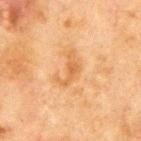The lesion was photographed on a routine skin check and not biopsied; there is no pathology result.
The lesion is on the chest.
Automated image analysis of the tile measured an eccentricity of roughly 0.85 and a symmetry-axis asymmetry near 0.7. And it measured a lesion color around L≈51 a*≈20 b*≈37 in CIELAB, a lesion–skin lightness drop of about 7, and a normalized lesion–skin contrast near 6. The software also gave a border-irregularity rating of about 8.5/10, a color-variation rating of about 2/10, and radial color variation of about 1.
The patient is a male about 65 years old.
The recorded lesion diameter is about 4 mm.
This is a cross-polarized tile.
A 15 mm close-up tile from a total-body photography series done for melanoma screening.